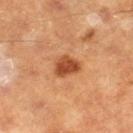The lesion was tiled from a total-body skin photograph and was not biopsied. The recorded lesion diameter is about 3.5 mm. Imaged with cross-polarized lighting. A roughly 15 mm field-of-view crop from a total-body skin photograph. The subject is a male aged 58–62. From the leg.